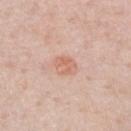Impression: Imaged during a routine full-body skin examination; the lesion was not biopsied and no histopathology is available. Acquisition and patient details: A 15 mm close-up extracted from a 3D total-body photography capture. A male subject about 60 years old. The lesion is located on the chest. Captured under white-light illumination.